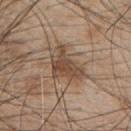| field | value |
|---|---|
| notes | catalogued during a skin exam; not biopsied |
| subject | male, aged 48 to 52 |
| size | about 5 mm |
| body site | the chest |
| acquisition | ~15 mm tile from a whole-body skin photo |
| automated metrics | a shape-asymmetry score of about 0.45 (0 = symmetric); an average lesion color of about L≈47 a*≈16 b*≈28 (CIELAB) and a normalized lesion–skin contrast near 8; a border-irregularity rating of about 5/10 and radial color variation of about 1.5 |
| tile lighting | white-light |